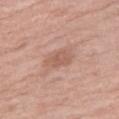A 15 mm close-up extracted from a 3D total-body photography capture.
An algorithmic analysis of the crop reported a mean CIELAB color near L≈57 a*≈21 b*≈28, a lesion–skin lightness drop of about 8, and a normalized border contrast of about 5.5. The software also gave an automated nevus-likeness rating near 5 out of 100 and a detector confidence of about 100 out of 100 that the crop contains a lesion.
Captured under white-light illumination.
A female subject about 70 years old.
Located on the leg.
Measured at roughly 3 mm in maximum diameter.